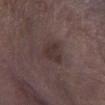Q: Is there a histopathology result?
A: imaged on a skin check; not biopsied
Q: What did automated image analysis measure?
A: an area of roughly 6 mm² and a shape-asymmetry score of about 0.3 (0 = symmetric); a mean CIELAB color near L≈32 a*≈14 b*≈16; internal color variation of about 2.5 on a 0–10 scale and radial color variation of about 1
Q: What is the imaging modality?
A: ~15 mm crop, total-body skin-cancer survey
Q: What is the anatomic site?
A: the leg
Q: Illumination type?
A: white-light illumination
Q: Patient demographics?
A: male, aged approximately 70